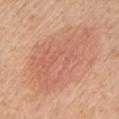The lesion was photographed on a routine skin check and not biopsied; there is no pathology result.
A roughly 15 mm field-of-view crop from a total-body skin photograph.
A female patient, in their 50s.
On the left upper arm.